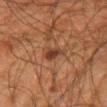Impression: This lesion was catalogued during total-body skin photography and was not selected for biopsy. Image and clinical context: This image is a 15 mm lesion crop taken from a total-body photograph. The total-body-photography lesion software estimated lesion-presence confidence of about 100/100. The subject is a male in their mid- to late 60s. The lesion's longest dimension is about 3 mm. This is a cross-polarized tile. From the right upper arm.